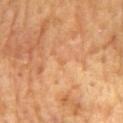Clinical impression:
The lesion was photographed on a routine skin check and not biopsied; there is no pathology result.
Background:
From the mid back. A 15 mm close-up tile from a total-body photography series done for melanoma screening. The lesion-visualizer software estimated a footprint of about 1 mm², an eccentricity of roughly 0.75, and two-axis asymmetry of about 0.3. The software also gave a lesion color around L≈61 a*≈23 b*≈40 in CIELAB and roughly 4 lightness units darker than nearby skin. It also reported a border-irregularity rating of about 2.5/10, internal color variation of about 0 on a 0–10 scale, and radial color variation of about 0. About 1 mm across. The subject is a male roughly 60 years of age. The tile uses cross-polarized illumination.